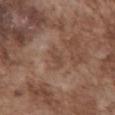Assessment:
This lesion was catalogued during total-body skin photography and was not selected for biopsy.
Background:
Imaged with white-light lighting. A roughly 15 mm field-of-view crop from a total-body skin photograph. The recorded lesion diameter is about 2.5 mm. A male patient, aged approximately 75. From the abdomen.